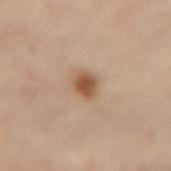Assessment: The lesion was photographed on a routine skin check and not biopsied; there is no pathology result. Clinical summary: The tile uses cross-polarized illumination. A close-up tile cropped from a whole-body skin photograph, about 15 mm across. The lesion is located on the lower back. The patient is a female approximately 60 years of age.